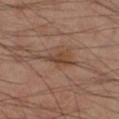biopsy_status: not biopsied; imaged during a skin examination
site: left lower leg
automated_metrics:
  border_irregularity_0_10: 4.5
  color_variation_0_10: 2.5
  nevus_likeness_0_100: 75
  lesion_detection_confidence_0_100: 100
patient:
  sex: male
  age_approx: 50
lesion_size:
  long_diameter_mm_approx: 3.5
lighting: cross-polarized
image:
  source: total-body photography crop
  field_of_view_mm: 15